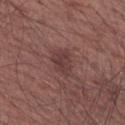site: right upper arm
image:
  source: total-body photography crop
  field_of_view_mm: 15
patient:
  sex: male
  age_approx: 65